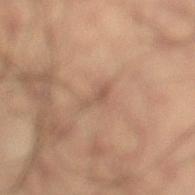| feature | finding |
|---|---|
| workup | imaged on a skin check; not biopsied |
| imaging modality | total-body-photography crop, ~15 mm field of view |
| tile lighting | cross-polarized |
| size | ≈3 mm |
| patient | male, aged 33–37 |
| location | the left lower leg |